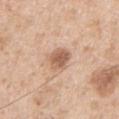No biopsy was performed on this lesion — it was imaged during a full skin examination and was not determined to be concerning. From the left upper arm. A 15 mm close-up tile from a total-body photography series done for melanoma screening. Approximately 3 mm at its widest. A male patient roughly 55 years of age.